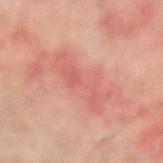illumination = cross-polarized
TBP lesion metrics = a footprint of about 12 mm² and a shape eccentricity near 0.95; a border-irregularity index near 8.5/10, internal color variation of about 2 on a 0–10 scale, and a peripheral color-asymmetry measure near 0.5
patient = male, aged approximately 60
image source = ~15 mm tile from a whole-body skin photo
location = the left forearm
lesion size = ~7 mm (longest diameter)